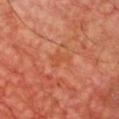The lesion was photographed on a routine skin check and not biopsied; there is no pathology result.
The recorded lesion diameter is about 3 mm.
A male patient aged 63 to 67.
From the chest.
The lesion-visualizer software estimated border irregularity of about 4.5 on a 0–10 scale.
A 15 mm close-up extracted from a 3D total-body photography capture.
Captured under cross-polarized illumination.